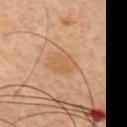Recorded during total-body skin imaging; not selected for excision or biopsy.
A 15 mm close-up extracted from a 3D total-body photography capture.
The subject is a male aged 43–47.
The lesion-visualizer software estimated a lesion area of about 7 mm², a shape eccentricity near 0.55, and two-axis asymmetry of about 0.2. The software also gave a mean CIELAB color near L≈55 a*≈19 b*≈35 and a lesion–skin lightness drop of about 6. And it measured an automated nevus-likeness rating near 20 out of 100 and a detector confidence of about 100 out of 100 that the crop contains a lesion.
On the chest.